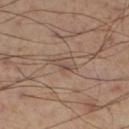notes: catalogued during a skin exam; not biopsied
subject: male, aged around 55
size: ≈3 mm
illumination: cross-polarized illumination
image source: ~15 mm crop, total-body skin-cancer survey
body site: the right lower leg